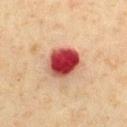Q: What kind of image is this?
A: 15 mm crop, total-body photography
Q: What did automated image analysis measure?
A: an area of roughly 13 mm², an eccentricity of roughly 0.45, and two-axis asymmetry of about 0.1; an average lesion color of about L≈43 a*≈33 b*≈27 (CIELAB), roughly 21 lightness units darker than nearby skin, and a lesion-to-skin contrast of about 14.5 (normalized; higher = more distinct); an automated nevus-likeness rating near 0 out of 100 and a lesion-detection confidence of about 100/100
Q: Where on the body is the lesion?
A: the chest
Q: What lighting was used for the tile?
A: cross-polarized
Q: Lesion size?
A: ~4 mm (longest diameter)
Q: Who is the patient?
A: male, approximately 60 years of age